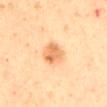The lesion is on the back.
The subject is a male aged 28–32.
A 15 mm crop from a total-body photograph taken for skin-cancer surveillance.
The tile uses cross-polarized illumination.
Measured at roughly 3.5 mm in maximum diameter.